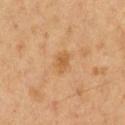Assessment: No biopsy was performed on this lesion — it was imaged during a full skin examination and was not determined to be concerning. Image and clinical context: Located on the chest. Cropped from a whole-body photographic skin survey; the tile spans about 15 mm. A male patient, aged around 50. Imaged with cross-polarized lighting. About 2.5 mm across.